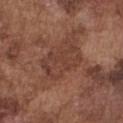Imaged during a routine full-body skin examination; the lesion was not biopsied and no histopathology is available. The subject is a male aged 73–77. Imaged with white-light lighting. Automated tile analysis of the lesion measured a lesion color around L≈40 a*≈21 b*≈26 in CIELAB. It also reported a nevus-likeness score of about 5/100 and a detector confidence of about 85 out of 100 that the crop contains a lesion. On the front of the torso. A region of skin cropped from a whole-body photographic capture, roughly 15 mm wide.